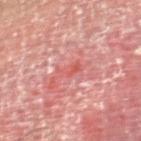Impression:
Imaged during a routine full-body skin examination; the lesion was not biopsied and no histopathology is available.
Clinical summary:
A male subject approximately 55 years of age. An algorithmic analysis of the crop reported a shape eccentricity near 0.8 and two-axis asymmetry of about 0.3. And it measured a border-irregularity rating of about 3.5/10, a color-variation rating of about 3/10, and radial color variation of about 1. And it measured an automated nevus-likeness rating near 0 out of 100 and a lesion-detection confidence of about 75/100. On the right thigh. This is a cross-polarized tile. A lesion tile, about 15 mm wide, cut from a 3D total-body photograph. Measured at roughly 3 mm in maximum diameter.